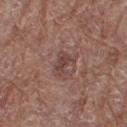A 15 mm crop from a total-body photograph taken for skin-cancer surveillance. A female subject, in their 80s. An algorithmic analysis of the crop reported a lesion area of about 4 mm², a shape eccentricity near 0.7, and two-axis asymmetry of about 0.4. And it measured a mean CIELAB color near L≈42 a*≈19 b*≈22, a lesion–skin lightness drop of about 8, and a normalized lesion–skin contrast near 7. The analysis additionally found a border-irregularity index near 4.5/10 and a peripheral color-asymmetry measure near 0.5. And it measured an automated nevus-likeness rating near 0 out of 100 and lesion-presence confidence of about 100/100. This is a white-light tile. On the right thigh. The lesion's longest dimension is about 3 mm.